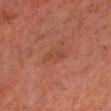{
  "patient": {
    "sex": "male",
    "age_approx": 60
  },
  "automated_metrics": {
    "area_mm2_approx": 3.5,
    "eccentricity": 0.9,
    "shape_asymmetry": 0.2,
    "vs_skin_darker_L": 5.0,
    "border_irregularity_0_10": 2.5,
    "color_variation_0_10": 2.5,
    "nevus_likeness_0_100": 0,
    "lesion_detection_confidence_0_100": 100
  },
  "lighting": "cross-polarized",
  "lesion_size": {
    "long_diameter_mm_approx": 3.0
  },
  "site": "head or neck",
  "image": {
    "source": "total-body photography crop",
    "field_of_view_mm": 15
  }
}